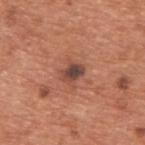Clinical impression:
No biopsy was performed on this lesion — it was imaged during a full skin examination and was not determined to be concerning.
Image and clinical context:
The lesion is on the upper back. The tile uses white-light illumination. Measured at roughly 3 mm in maximum diameter. A male patient aged approximately 65. A 15 mm close-up extracted from a 3D total-body photography capture.